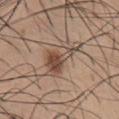<tbp_lesion>
  <biopsy_status>not biopsied; imaged during a skin examination</biopsy_status>
  <patient>
    <sex>male</sex>
    <age_approx>20</age_approx>
  </patient>
  <site>chest</site>
  <automated_metrics>
    <area_mm2_approx>10.0</area_mm2_approx>
    <eccentricity>0.9</eccentricity>
    <shape_asymmetry>0.7</shape_asymmetry>
    <cielab_L>49</cielab_L>
    <cielab_a>17</cielab_a>
    <cielab_b>26</cielab_b>
    <vs_skin_darker_L>11.0</vs_skin_darker_L>
    <border_irregularity_0_10>8.0</border_irregularity_0_10>
    <color_variation_0_10>4.0</color_variation_0_10>
    <peripheral_color_asymmetry>1.0</peripheral_color_asymmetry>
    <nevus_likeness_0_100>100</nevus_likeness_0_100>
    <lesion_detection_confidence_0_100>100</lesion_detection_confidence_0_100>
  </automated_metrics>
  <image>
    <source>total-body photography crop</source>
    <field_of_view_mm>15</field_of_view_mm>
  </image>
  <lighting>white-light</lighting>
  <lesion_size>
    <long_diameter_mm_approx>6.0</long_diameter_mm_approx>
  </lesion_size>
</tbp_lesion>